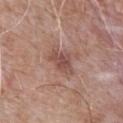The lesion was tiled from a total-body skin photograph and was not biopsied.